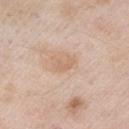Clinical impression: The lesion was photographed on a routine skin check and not biopsied; there is no pathology result. Acquisition and patient details: A male patient, aged 53–57. On the arm. Cropped from a total-body skin-imaging series; the visible field is about 15 mm. The recorded lesion diameter is about 2.5 mm.